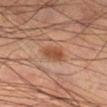This lesion was catalogued during total-body skin photography and was not selected for biopsy.
Captured under cross-polarized illumination.
Automated image analysis of the tile measured border irregularity of about 2 on a 0–10 scale and a peripheral color-asymmetry measure near 1.5.
Cropped from a total-body skin-imaging series; the visible field is about 15 mm.
Longest diameter approximately 3 mm.
The lesion is located on the right lower leg.
The patient is a male roughly 45 years of age.